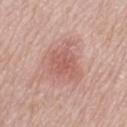Assessment: This lesion was catalogued during total-body skin photography and was not selected for biopsy. Context: This is a white-light tile. A lesion tile, about 15 mm wide, cut from a 3D total-body photograph. On the leg. The recorded lesion diameter is about 3.5 mm. A female patient aged 58–62.